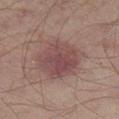Case summary:
– workup · no biopsy performed (imaged during a skin exam)